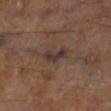{
  "biopsy_status": "not biopsied; imaged during a skin examination",
  "lesion_size": {
    "long_diameter_mm_approx": 3.5
  },
  "image": {
    "source": "total-body photography crop",
    "field_of_view_mm": 15
  },
  "lighting": "cross-polarized",
  "site": "leg"
}